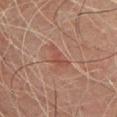Findings:
– patient · male, aged 43–47
– anatomic site · the chest
– image source · ~15 mm tile from a whole-body skin photo